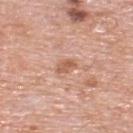Q: Is there a histopathology result?
A: total-body-photography surveillance lesion; no biopsy
Q: How large is the lesion?
A: about 2.5 mm
Q: What kind of image is this?
A: ~15 mm crop, total-body skin-cancer survey
Q: What lighting was used for the tile?
A: white-light illumination
Q: What are the patient's age and sex?
A: male, aged 78 to 82
Q: What did automated image analysis measure?
A: a lesion area of about 3 mm²; a border-irregularity index near 3/10 and internal color variation of about 2 on a 0–10 scale; a nevus-likeness score of about 0/100 and a detector confidence of about 100 out of 100 that the crop contains a lesion
Q: Lesion location?
A: the upper back